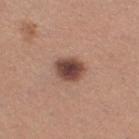Assessment: Recorded during total-body skin imaging; not selected for excision or biopsy.